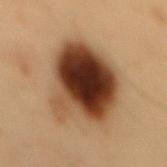follow-up: no biopsy performed (imaged during a skin exam) | image source: 15 mm crop, total-body photography | lighting: cross-polarized | subject: male, approximately 55 years of age | size: about 9 mm | anatomic site: the mid back.